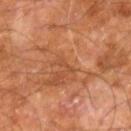• subject · male, about 60 years old
• anatomic site · the right leg
• size · ~2.5 mm (longest diameter)
• tile lighting · cross-polarized
• image source · ~15 mm tile from a whole-body skin photo